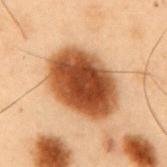Assessment: Imaged during a routine full-body skin examination; the lesion was not biopsied and no histopathology is available. Acquisition and patient details: The subject is a male approximately 55 years of age. This image is a 15 mm lesion crop taken from a total-body photograph. From the mid back.